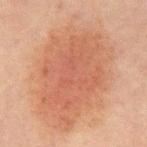The lesion was tiled from a total-body skin photograph and was not biopsied.
The lesion is located on the mid back.
A male patient about 65 years old.
Cropped from a whole-body photographic skin survey; the tile spans about 15 mm.
About 13 mm across.
The tile uses cross-polarized illumination.